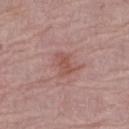Notes:
- notes: total-body-photography surveillance lesion; no biopsy
- imaging modality: 15 mm crop, total-body photography
- patient: female, in their mid- to late 60s
- site: the leg